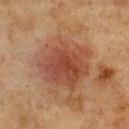<lesion>
<biopsy_status>not biopsied; imaged during a skin examination</biopsy_status>
<lighting>cross-polarized</lighting>
<image>
  <source>total-body photography crop</source>
  <field_of_view_mm>15</field_of_view_mm>
</image>
<lesion_size>
  <long_diameter_mm_approx>6.0</long_diameter_mm_approx>
</lesion_size>
<site>chest</site>
<patient>
  <sex>male</sex>
  <age_approx>75</age_approx>
</patient>
</lesion>